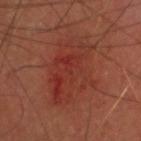biopsy_status: not biopsied; imaged during a skin examination
site: head or neck
patient:
  sex: male
  age_approx: 65
automated_metrics:
  area_mm2_approx: 28.0
  eccentricity: 0.8
  shape_asymmetry: 0.25
  border_irregularity_0_10: 5.5
  peripheral_color_asymmetry: 2.5
  lesion_detection_confidence_0_100: 100
lesion_size:
  long_diameter_mm_approx: 7.5
image:
  source: total-body photography crop
  field_of_view_mm: 15